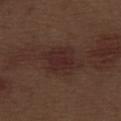Captured during whole-body skin photography for melanoma surveillance; the lesion was not biopsied. This image is a 15 mm lesion crop taken from a total-body photograph. The lesion's longest dimension is about 5 mm. Captured under white-light illumination. The patient is a male in their 70s. From the abdomen.